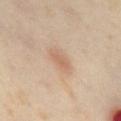Notes:
– notes: imaged on a skin check; not biopsied
– imaging modality: 15 mm crop, total-body photography
– body site: the mid back
– subject: female, about 55 years old
– automated lesion analysis: border irregularity of about 3 on a 0–10 scale, a within-lesion color-variation index near 2/10, and a peripheral color-asymmetry measure near 0.5
– diameter: about 3 mm
– tile lighting: cross-polarized illumination